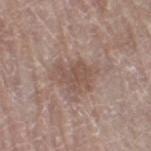Clinical summary:
This is a white-light tile. A female subject aged 58 to 62. About 3.5 mm across. A roughly 15 mm field-of-view crop from a total-body skin photograph. Automated image analysis of the tile measured a footprint of about 7 mm² and a shape eccentricity near 0.65. It also reported a lesion color around L≈51 a*≈17 b*≈24 in CIELAB and about 8 CIELAB-L* units darker than the surrounding skin. The analysis additionally found a border-irregularity index near 5/10 and a peripheral color-asymmetry measure near 0.5. And it measured a detector confidence of about 100 out of 100 that the crop contains a lesion. From the right thigh.